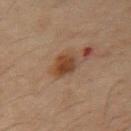Clinical impression:
The lesion was photographed on a routine skin check and not biopsied; there is no pathology result.
Background:
Automated image analysis of the tile measured an area of roughly 5 mm², an eccentricity of roughly 0.65, and two-axis asymmetry of about 0.2. It also reported an automated nevus-likeness rating near 100 out of 100 and a detector confidence of about 100 out of 100 that the crop contains a lesion. A male patient, in their 50s. Cropped from a whole-body photographic skin survey; the tile spans about 15 mm. From the abdomen. Longest diameter approximately 2.5 mm.